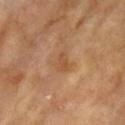This lesion was catalogued during total-body skin photography and was not selected for biopsy. Imaged with cross-polarized lighting. A 15 mm close-up extracted from a 3D total-body photography capture. Approximately 2.5 mm at its widest. From the right upper arm. A female patient aged 73 to 77.